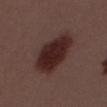Q: What is the lesion's diameter?
A: ~6.5 mm (longest diameter)
Q: Patient demographics?
A: male, approximately 55 years of age
Q: Lesion location?
A: the mid back
Q: How was this image acquired?
A: 15 mm crop, total-body photography
Q: What did automated image analysis measure?
A: border irregularity of about 2 on a 0–10 scale, internal color variation of about 3 on a 0–10 scale, and a peripheral color-asymmetry measure near 1